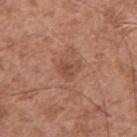The lesion was tiled from a total-body skin photograph and was not biopsied. A male subject, aged 48 to 52. A 15 mm close-up extracted from a 3D total-body photography capture. The lesion is on the right upper arm. Captured under white-light illumination.